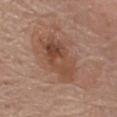– follow-up — no biopsy performed (imaged during a skin exam)
– body site — the chest
– lesion size — about 7 mm
– imaging modality — ~15 mm crop, total-body skin-cancer survey
– patient — male, roughly 70 years of age
– image-analysis metrics — a footprint of about 18 mm² and a symmetry-axis asymmetry near 0.25; a nevus-likeness score of about 65/100 and a lesion-detection confidence of about 100/100
– illumination — white-light illumination